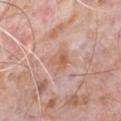The lesion is located on the chest. A region of skin cropped from a whole-body photographic capture, roughly 15 mm wide. A male subject aged approximately 80. The tile uses white-light illumination. Automated image analysis of the tile measured a shape eccentricity near 0.4 and a symmetry-axis asymmetry near 0.25. The software also gave roughly 8 lightness units darker than nearby skin and a normalized lesion–skin contrast near 6.5. And it measured internal color variation of about 4.5 on a 0–10 scale and radial color variation of about 1.5. Approximately 2.5 mm at its widest.